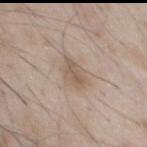Findings:
* acquisition: ~15 mm crop, total-body skin-cancer survey
* body site: the front of the torso
* tile lighting: white-light
* patient: male, aged around 50